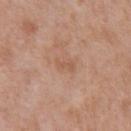Assessment:
The lesion was tiled from a total-body skin photograph and was not biopsied.
Context:
About 2.5 mm across. An algorithmic analysis of the crop reported a footprint of about 3 mm², a shape eccentricity near 0.9, and two-axis asymmetry of about 0.25. And it measured a nevus-likeness score of about 0/100. The lesion is located on the chest. A region of skin cropped from a whole-body photographic capture, roughly 15 mm wide. This is a white-light tile. A male patient aged 68–72.